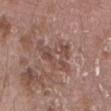The lesion was tiled from a total-body skin photograph and was not biopsied. The patient is a male approximately 55 years of age. The lesion is on the lower back. The lesion's longest dimension is about 5 mm. A roughly 15 mm field-of-view crop from a total-body skin photograph. Captured under white-light illumination.